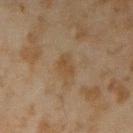The lesion was photographed on a routine skin check and not biopsied; there is no pathology result. A male subject approximately 45 years of age. This is a cross-polarized tile. The total-body-photography lesion software estimated a footprint of about 4.5 mm², an eccentricity of roughly 0.75, and a shape-asymmetry score of about 0.2 (0 = symmetric). It also reported an average lesion color of about L≈38 a*≈12 b*≈28 (CIELAB), a lesion–skin lightness drop of about 5, and a normalized border contrast of about 5.5. The analysis additionally found border irregularity of about 2 on a 0–10 scale, internal color variation of about 1.5 on a 0–10 scale, and a peripheral color-asymmetry measure near 0.5. Located on the left forearm. A 15 mm close-up extracted from a 3D total-body photography capture.